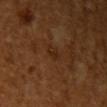workup: no biopsy performed (imaged during a skin exam) | subject: female, about 55 years old | anatomic site: the right upper arm | lesion size: about 2 mm | automated metrics: a shape eccentricity near 0.7 and a shape-asymmetry score of about 0.2 (0 = symmetric); border irregularity of about 1.5 on a 0–10 scale, a color-variation rating of about 0.5/10, and radial color variation of about 0; a classifier nevus-likeness of about 0/100 and lesion-presence confidence of about 100/100 | image: ~15 mm crop, total-body skin-cancer survey | illumination: cross-polarized illumination.